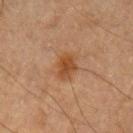Clinical impression:
The lesion was photographed on a routine skin check and not biopsied; there is no pathology result.
Background:
A male subject aged 63 to 67. Captured under cross-polarized illumination. Automated tile analysis of the lesion measured a footprint of about 5 mm², an eccentricity of roughly 0.7, and a shape-asymmetry score of about 0.25 (0 = symmetric). The analysis additionally found about 9 CIELAB-L* units darker than the surrounding skin and a lesion-to-skin contrast of about 8.5 (normalized; higher = more distinct). The software also gave a border-irregularity rating of about 2.5/10, internal color variation of about 2.5 on a 0–10 scale, and radial color variation of about 1. Located on the arm. A close-up tile cropped from a whole-body skin photograph, about 15 mm across. About 3 mm across.